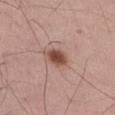lighting: white-light
image:
  source: total-body photography crop
  field_of_view_mm: 15
automated_metrics:
  cielab_L: 48
  cielab_a: 22
  cielab_b: 26
  vs_skin_darker_L: 14.0
  vs_skin_contrast_norm: 10.0
  border_irregularity_0_10: 2.0
  color_variation_0_10: 2.5
  peripheral_color_asymmetry: 0.5
  nevus_likeness_0_100: 100
  lesion_detection_confidence_0_100: 100
lesion_size:
  long_diameter_mm_approx: 2.5
site: left thigh
patient:
  sex: male
  age_approx: 55